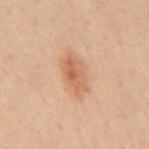Imaged during a routine full-body skin examination; the lesion was not biopsied and no histopathology is available. Measured at roughly 4.5 mm in maximum diameter. A male patient, approximately 50 years of age. Located on the mid back. A close-up tile cropped from a whole-body skin photograph, about 15 mm across. This is a cross-polarized tile.